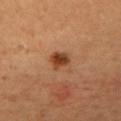* biopsy status · imaged on a skin check; not biopsied
* subject · female, approximately 30 years of age
* illumination · cross-polarized illumination
* acquisition · ~15 mm crop, total-body skin-cancer survey
* location · the chest
* lesion diameter · about 2.5 mm